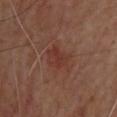Q: Is there a histopathology result?
A: no biopsy performed (imaged during a skin exam)
Q: Lesion location?
A: the upper back
Q: What is the lesion's diameter?
A: about 4.5 mm
Q: What is the imaging modality?
A: ~15 mm crop, total-body skin-cancer survey
Q: Who is the patient?
A: male, in their mid-60s
Q: How was the tile lit?
A: cross-polarized illumination